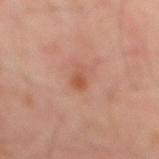Context: The recorded lesion diameter is about 2.5 mm. Imaged with cross-polarized lighting. The lesion-visualizer software estimated a footprint of about 3 mm², an eccentricity of roughly 0.8, and two-axis asymmetry of about 0.3. It also reported a lesion color around L≈53 a*≈25 b*≈32 in CIELAB, about 8 CIELAB-L* units darker than the surrounding skin, and a lesion-to-skin contrast of about 6.5 (normalized; higher = more distinct). The analysis additionally found border irregularity of about 3 on a 0–10 scale, a color-variation rating of about 1.5/10, and peripheral color asymmetry of about 0.5. The analysis additionally found a classifier nevus-likeness of about 20/100 and a lesion-detection confidence of about 100/100. A male patient, aged around 50. A 15 mm close-up tile from a total-body photography series done for melanoma screening. The lesion is on the mid back.